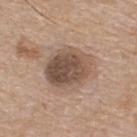Assessment: No biopsy was performed on this lesion — it was imaged during a full skin examination and was not determined to be concerning. Context: The lesion is on the back. A 15 mm crop from a total-body photograph taken for skin-cancer surveillance. Captured under white-light illumination. A male patient aged approximately 75. The recorded lesion diameter is about 5.5 mm.